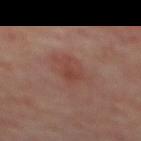The lesion was tiled from a total-body skin photograph and was not biopsied. Located on the mid back. Automated tile analysis of the lesion measured a border-irregularity rating of about 3.5/10, a color-variation rating of about 3/10, and a peripheral color-asymmetry measure near 1. The patient is a male about 70 years old. This is a cross-polarized tile. A 15 mm close-up extracted from a 3D total-body photography capture.